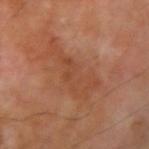biopsy status: total-body-photography surveillance lesion; no biopsy
acquisition: ~15 mm crop, total-body skin-cancer survey
patient: male, aged 68 to 72
size: about 6.5 mm
site: the right upper arm